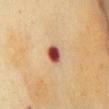Impression: The lesion was tiled from a total-body skin photograph and was not biopsied. Context: Located on the chest. The lesion's longest dimension is about 3 mm. A region of skin cropped from a whole-body photographic capture, roughly 15 mm wide. The tile uses cross-polarized illumination. A female patient, aged 58 to 62.